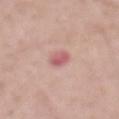The lesion was photographed on a routine skin check and not biopsied; there is no pathology result. The total-body-photography lesion software estimated a lesion area of about 4 mm², an outline eccentricity of about 0.75 (0 = round, 1 = elongated), and a shape-asymmetry score of about 0.15 (0 = symmetric). The lesion is on the abdomen. Approximately 2.5 mm at its widest. A male patient, aged 48–52. A 15 mm close-up tile from a total-body photography series done for melanoma screening. The tile uses white-light illumination.